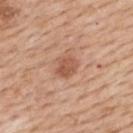Recorded during total-body skin imaging; not selected for excision or biopsy.